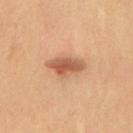Q: Was a biopsy performed?
A: no biopsy performed (imaged during a skin exam)
Q: What is the imaging modality?
A: ~15 mm tile from a whole-body skin photo
Q: What is the anatomic site?
A: the chest
Q: What are the patient's age and sex?
A: male, aged 68–72